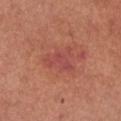workup = catalogued during a skin exam; not biopsied | acquisition = total-body-photography crop, ~15 mm field of view | illumination = white-light illumination | subject = female, aged around 65 | site = the chest | diameter = ~4 mm (longest diameter).